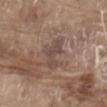Part of a total-body skin-imaging series; this lesion was reviewed on a skin check and was not flagged for biopsy. A male patient aged approximately 80. Captured under white-light illumination. The lesion is on the abdomen. Longest diameter approximately 4 mm. Cropped from a whole-body photographic skin survey; the tile spans about 15 mm. Automated image analysis of the tile measured roughly 7 lightness units darker than nearby skin and a normalized lesion–skin contrast near 6.